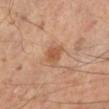workup: catalogued during a skin exam; not biopsied | acquisition: ~15 mm crop, total-body skin-cancer survey | tile lighting: cross-polarized | automated metrics: an average lesion color of about L≈53 a*≈23 b*≈35 (CIELAB), about 9 CIELAB-L* units darker than the surrounding skin, and a normalized border contrast of about 7; a border-irregularity index near 2/10 and radial color variation of about 0.5 | size: ≈2 mm | subject: male, approximately 55 years of age | site: the left lower leg.